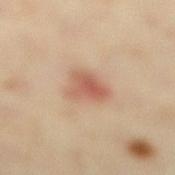Recorded during total-body skin imaging; not selected for excision or biopsy. Automated tile analysis of the lesion measured a mean CIELAB color near L≈55 a*≈22 b*≈30, a lesion–skin lightness drop of about 11, and a lesion-to-skin contrast of about 7 (normalized; higher = more distinct). And it measured internal color variation of about 4.5 on a 0–10 scale and a peripheral color-asymmetry measure near 1.5. It also reported an automated nevus-likeness rating near 90 out of 100 and lesion-presence confidence of about 100/100. A lesion tile, about 15 mm wide, cut from a 3D total-body photograph. The patient is a female aged approximately 35. On the right lower leg. Imaged with cross-polarized lighting.